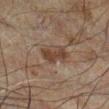<lesion>
<automated_metrics>
  <nevus_likeness_0_100>15</nevus_likeness_0_100>
</automated_metrics>
<lighting>cross-polarized</lighting>
<patient>
  <sex>male</sex>
  <age_approx>60</age_approx>
</patient>
<site>left leg</site>
<image>
  <source>total-body photography crop</source>
  <field_of_view_mm>15</field_of_view_mm>
</image>
<lesion_size>
  <long_diameter_mm_approx>3.5</long_diameter_mm_approx>
</lesion_size>
</lesion>